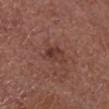Part of a total-body skin-imaging series; this lesion was reviewed on a skin check and was not flagged for biopsy. Cropped from a total-body skin-imaging series; the visible field is about 15 mm. A male patient in their 70s. From the head or neck.